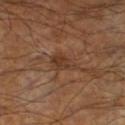Captured under cross-polarized illumination. The lesion is on the leg. The total-body-photography lesion software estimated a shape eccentricity near 0.75 and a symmetry-axis asymmetry near 0.35. And it measured a lesion color around L≈35 a*≈19 b*≈28 in CIELAB, roughly 7 lightness units darker than nearby skin, and a normalized lesion–skin contrast near 6.5. The software also gave border irregularity of about 3.5 on a 0–10 scale, internal color variation of about 3.5 on a 0–10 scale, and peripheral color asymmetry of about 1. The analysis additionally found a classifier nevus-likeness of about 0/100 and a lesion-detection confidence of about 95/100. This image is a 15 mm lesion crop taken from a total-body photograph. The subject is a male in their 60s. The lesion's longest dimension is about 3.5 mm.